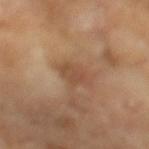workup: no biopsy performed (imaged during a skin exam); subject: male, aged around 65; location: the left lower leg; size: about 3 mm; acquisition: 15 mm crop, total-body photography.